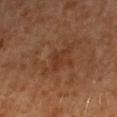<case>
  <biopsy_status>not biopsied; imaged during a skin examination</biopsy_status>
  <automated_metrics>
    <area_mm2_approx>3.5</area_mm2_approx>
    <cielab_L>34</cielab_L>
    <cielab_a>22</cielab_a>
    <cielab_b>30</cielab_b>
    <vs_skin_darker_L>5.0</vs_skin_darker_L>
    <border_irregularity_0_10>6.0</border_irregularity_0_10>
    <color_variation_0_10>0.5</color_variation_0_10>
  </automated_metrics>
  <image>
    <source>total-body photography crop</source>
    <field_of_view_mm>15</field_of_view_mm>
  </image>
  <site>right forearm</site>
  <lighting>cross-polarized</lighting>
  <patient>
    <sex>female</sex>
    <age_approx>65</age_approx>
  </patient>
</case>